From the left lower leg; the tile uses cross-polarized illumination; the recorded lesion diameter is about 5 mm; cropped from a whole-body photographic skin survey; the tile spans about 15 mm; a female subject, aged 73 to 77.
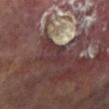histopathologic diagnosis=an actinic keratosis — an indeterminate (borderline) lesion.